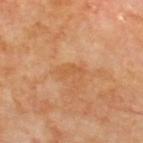<case>
<biopsy_status>not biopsied; imaged during a skin examination</biopsy_status>
<lighting>cross-polarized</lighting>
<image>
  <source>total-body photography crop</source>
  <field_of_view_mm>15</field_of_view_mm>
</image>
<patient>
  <sex>male</sex>
  <age_approx>70</age_approx>
</patient>
<automated_metrics>
  <shape_asymmetry>0.45</shape_asymmetry>
  <border_irregularity_0_10>5.0</border_irregularity_0_10>
  <nevus_likeness_0_100>0</nevus_likeness_0_100>
  <lesion_detection_confidence_0_100>100</lesion_detection_confidence_0_100>
</automated_metrics>
<site>upper back</site>
</case>